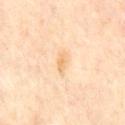follow-up = total-body-photography surveillance lesion; no biopsy | subject = male, about 65 years old | lesion size = ~3 mm (longest diameter) | lighting = cross-polarized | anatomic site = the chest | image = ~15 mm crop, total-body skin-cancer survey.